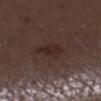Notes:
* follow-up — total-body-photography surveillance lesion; no biopsy
* anatomic site — the right lower leg
* lesion diameter — ≈3.5 mm
* illumination — white-light illumination
* TBP lesion metrics — an outline eccentricity of about 0.8 (0 = round, 1 = elongated) and two-axis asymmetry of about 0.3; an average lesion color of about L≈25 a*≈15 b*≈18 (CIELAB) and a lesion–skin lightness drop of about 6; lesion-presence confidence of about 100/100
* subject — female, aged 48–52
* acquisition — ~15 mm tile from a whole-body skin photo